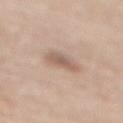{"biopsy_status": "not biopsied; imaged during a skin examination", "site": "mid back", "lighting": "white-light", "patient": {"sex": "female", "age_approx": 65}, "image": {"source": "total-body photography crop", "field_of_view_mm": 15}, "automated_metrics": {"vs_skin_darker_L": 10.0, "vs_skin_contrast_norm": 6.5, "border_irregularity_0_10": 2.5, "color_variation_0_10": 2.5, "peripheral_color_asymmetry": 1.0}}